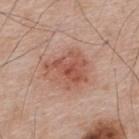workup = catalogued during a skin exam; not biopsied
image source = total-body-photography crop, ~15 mm field of view
site = the upper back
illumination = white-light illumination
patient = male, about 65 years old